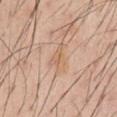From the chest. A close-up tile cropped from a whole-body skin photograph, about 15 mm across. Captured under white-light illumination. A male subject in their mid-40s. The recorded lesion diameter is about 3.5 mm.